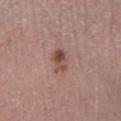Recorded during total-body skin imaging; not selected for excision or biopsy. A female patient in their mid-60s. On the left lower leg. The lesion-visualizer software estimated a symmetry-axis asymmetry near 0.3. The analysis additionally found a within-lesion color-variation index near 8.5/10 and peripheral color asymmetry of about 3.5. A 15 mm close-up extracted from a 3D total-body photography capture. Captured under white-light illumination. Measured at roughly 3 mm in maximum diameter.